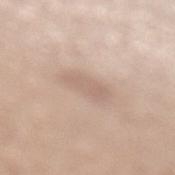This lesion was catalogued during total-body skin photography and was not selected for biopsy. A female subject, in their mid- to late 30s. On the left lower leg. A region of skin cropped from a whole-body photographic capture, roughly 15 mm wide. The lesion's longest dimension is about 4 mm. Captured under white-light illumination.